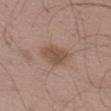Q: Is there a histopathology result?
A: total-body-photography surveillance lesion; no biopsy
Q: How was this image acquired?
A: ~15 mm tile from a whole-body skin photo
Q: What is the anatomic site?
A: the left thigh
Q: How was the tile lit?
A: white-light illumination
Q: What are the patient's age and sex?
A: male, about 50 years old